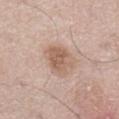On the left thigh. A male patient, in their mid- to late 70s. A roughly 15 mm field-of-view crop from a total-body skin photograph.